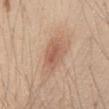workup: no biopsy performed (imaged during a skin exam)
subject: male, in their mid- to late 40s
acquisition: 15 mm crop, total-body photography
location: the abdomen